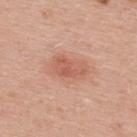Q: Is there a histopathology result?
A: no biopsy performed (imaged during a skin exam)
Q: How was the tile lit?
A: white-light
Q: How large is the lesion?
A: ~5 mm (longest diameter)
Q: What is the imaging modality?
A: 15 mm crop, total-body photography
Q: What are the patient's age and sex?
A: female, aged approximately 30
Q: Where on the body is the lesion?
A: the back